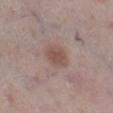<case>
<biopsy_status>not biopsied; imaged during a skin examination</biopsy_status>
</case>